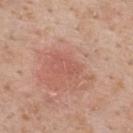| feature | finding |
|---|---|
| notes | no biopsy performed (imaged during a skin exam) |
| anatomic site | the back |
| automated metrics | a lesion area of about 4 mm², a shape eccentricity near 0.8, and two-axis asymmetry of about 0.4; a lesion color around L≈56 a*≈26 b*≈28 in CIELAB and a normalized border contrast of about 3.5; a border-irregularity rating of about 4/10 and radial color variation of about 1 |
| image | ~15 mm crop, total-body skin-cancer survey |
| patient | male, aged approximately 60 |
| size | about 3 mm |